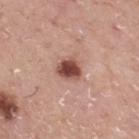The lesion was tiled from a total-body skin photograph and was not biopsied. This image is a 15 mm lesion crop taken from a total-body photograph. The tile uses white-light illumination. A male subject aged around 75. The lesion's longest dimension is about 3 mm. The total-body-photography lesion software estimated a footprint of about 5.5 mm², an eccentricity of roughly 0.5, and two-axis asymmetry of about 0.25. And it measured a normalized lesion–skin contrast near 12. The analysis additionally found border irregularity of about 2 on a 0–10 scale and a color-variation rating of about 3.5/10. The lesion is located on the mid back.